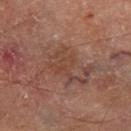Assessment: No biopsy was performed on this lesion — it was imaged during a full skin examination and was not determined to be concerning. Acquisition and patient details: Measured at roughly 5 mm in maximum diameter. Imaged with cross-polarized lighting. Located on the right thigh. A male subject aged 68 to 72. Cropped from a whole-body photographic skin survey; the tile spans about 15 mm.